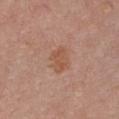<lesion>
  <biopsy_status>not biopsied; imaged during a skin examination</biopsy_status>
  <image>
    <source>total-body photography crop</source>
    <field_of_view_mm>15</field_of_view_mm>
  </image>
  <patient>
    <sex>female</sex>
    <age_approx>50</age_approx>
  </patient>
  <automated_metrics>
    <area_mm2_approx>5.0</area_mm2_approx>
    <shape_asymmetry>0.35</shape_asymmetry>
    <vs_skin_darker_L>7.0</vs_skin_darker_L>
    <vs_skin_contrast_norm>6.5</vs_skin_contrast_norm>
  </automated_metrics>
  <site>chest</site>
</lesion>